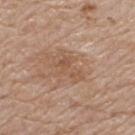Q: Was a biopsy performed?
A: catalogued during a skin exam; not biopsied
Q: What did automated image analysis measure?
A: a footprint of about 8 mm², a shape eccentricity near 0.8, and a symmetry-axis asymmetry near 0.4; a mean CIELAB color near L≈54 a*≈18 b*≈30, roughly 7 lightness units darker than nearby skin, and a normalized lesion–skin contrast near 5; a color-variation rating of about 3/10; a nevus-likeness score of about 0/100 and a lesion-detection confidence of about 100/100
Q: Lesion location?
A: the back
Q: Illumination type?
A: white-light illumination
Q: What kind of image is this?
A: total-body-photography crop, ~15 mm field of view
Q: What are the patient's age and sex?
A: male, aged 78–82